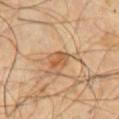Part of a total-body skin-imaging series; this lesion was reviewed on a skin check and was not flagged for biopsy.
Captured under cross-polarized illumination.
Automated image analysis of the tile measured a shape eccentricity near 0.75 and a shape-asymmetry score of about 0.25 (0 = symmetric). The analysis additionally found internal color variation of about 2.5 on a 0–10 scale and peripheral color asymmetry of about 0.5. The analysis additionally found a detector confidence of about 100 out of 100 that the crop contains a lesion.
A male patient aged around 55.
Measured at roughly 2.5 mm in maximum diameter.
This image is a 15 mm lesion crop taken from a total-body photograph.
From the chest.